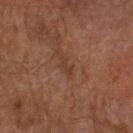Case summary:
– image source: 15 mm crop, total-body photography
– subject: male, in their mid- to late 70s
– location: the right forearm
– TBP lesion metrics: an average lesion color of about L≈31 a*≈16 b*≈24 (CIELAB), roughly 5 lightness units darker than nearby skin, and a normalized border contrast of about 5
– lighting: cross-polarized